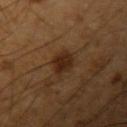- notes — total-body-photography surveillance lesion; no biopsy
- image-analysis metrics — a shape eccentricity near 0.35; a border-irregularity rating of about 2.5/10 and peripheral color asymmetry of about 1
- lighting — cross-polarized
- location — the left upper arm
- subject — male, about 65 years old
- acquisition — total-body-photography crop, ~15 mm field of view